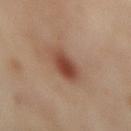{"biopsy_status": "not biopsied; imaged during a skin examination", "site": "mid back", "image": {"source": "total-body photography crop", "field_of_view_mm": 15}, "patient": {"sex": "female", "age_approx": 55}}